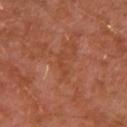Assessment:
The lesion was tiled from a total-body skin photograph and was not biopsied.
Acquisition and patient details:
Measured at roughly 3.5 mm in maximum diameter. The lesion is on the arm. Captured under cross-polarized illumination. A male subject, aged 28–32. A region of skin cropped from a whole-body photographic capture, roughly 15 mm wide.